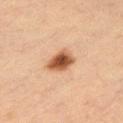workup = no biopsy performed (imaged during a skin exam).